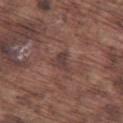Case summary:
– anatomic site: the left thigh
– patient: male, in their mid-70s
– lesion diameter: about 2.5 mm
– image source: total-body-photography crop, ~15 mm field of view
– lighting: white-light illumination
– automated metrics: a lesion color around L≈39 a*≈18 b*≈20 in CIELAB, about 7 CIELAB-L* units darker than the surrounding skin, and a normalized border contrast of about 7; a nevus-likeness score of about 0/100 and lesion-presence confidence of about 80/100